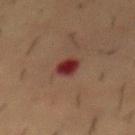Clinical impression: The lesion was tiled from a total-body skin photograph and was not biopsied. Acquisition and patient details: The subject is a male aged approximately 55. A region of skin cropped from a whole-body photographic capture, roughly 15 mm wide. From the mid back. The tile uses cross-polarized illumination. Automated image analysis of the tile measured a mean CIELAB color near L≈27 a*≈26 b*≈21, roughly 13 lightness units darker than nearby skin, and a lesion-to-skin contrast of about 12.5 (normalized; higher = more distinct). And it measured a border-irregularity rating of about 1.5/10, internal color variation of about 3.5 on a 0–10 scale, and radial color variation of about 1.